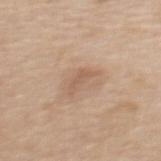biopsy status — no biopsy performed (imaged during a skin exam)
image — total-body-photography crop, ~15 mm field of view
illumination — white-light
anatomic site — the back
patient — female, aged approximately 65